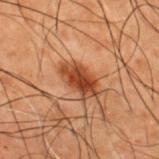| feature | finding |
|---|---|
| notes | total-body-photography surveillance lesion; no biopsy |
| patient | male, in their 50s |
| automated lesion analysis | a lesion area of about 8.5 mm² and two-axis asymmetry of about 0.2; border irregularity of about 2.5 on a 0–10 scale, internal color variation of about 4 on a 0–10 scale, and radial color variation of about 1.5; a classifier nevus-likeness of about 90/100 and lesion-presence confidence of about 100/100 |
| illumination | cross-polarized illumination |
| lesion diameter | ≈4.5 mm |
| site | the upper back |
| image source | ~15 mm crop, total-body skin-cancer survey |